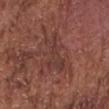Clinical impression: Part of a total-body skin-imaging series; this lesion was reviewed on a skin check and was not flagged for biopsy.